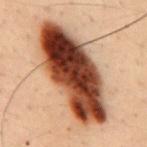Q: Was a biopsy performed?
A: no biopsy performed (imaged during a skin exam)
Q: How was this image acquired?
A: ~15 mm crop, total-body skin-cancer survey
Q: Where on the body is the lesion?
A: the back
Q: Patient demographics?
A: male, about 30 years old